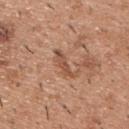workup = no biopsy performed (imaged during a skin exam) | subject = male, aged approximately 40 | acquisition = ~15 mm tile from a whole-body skin photo | illumination = white-light | TBP lesion metrics = an eccentricity of roughly 0.95 and two-axis asymmetry of about 0.45; a mean CIELAB color near L≈52 a*≈23 b*≈31, a lesion–skin lightness drop of about 9, and a normalized lesion–skin contrast near 6.5; border irregularity of about 5.5 on a 0–10 scale, internal color variation of about 1 on a 0–10 scale, and radial color variation of about 0 | size = about 4 mm | body site = the upper back.